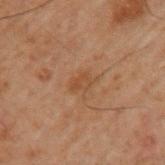Q: Was this lesion biopsied?
A: imaged on a skin check; not biopsied
Q: Where on the body is the lesion?
A: the left upper arm
Q: What is the imaging modality?
A: ~15 mm crop, total-body skin-cancer survey
Q: What is the lesion's diameter?
A: ~3 mm (longest diameter)
Q: How was the tile lit?
A: cross-polarized illumination
Q: Who is the patient?
A: male, approximately 60 years of age